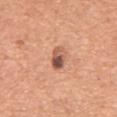Notes:
- biopsy status — no biopsy performed (imaged during a skin exam)